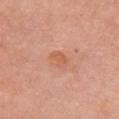The lesion was photographed on a routine skin check and not biopsied; there is no pathology result. From the chest. A region of skin cropped from a whole-body photographic capture, roughly 15 mm wide. The lesion's longest dimension is about 2.5 mm. Imaged with white-light lighting. The patient is a female approximately 75 years of age.